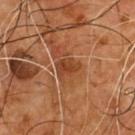{"biopsy_status": "not biopsied; imaged during a skin examination", "lighting": "cross-polarized", "image": {"source": "total-body photography crop", "field_of_view_mm": 15}, "lesion_size": {"long_diameter_mm_approx": 3.0}, "automated_metrics": {"vs_skin_darker_L": 9.0, "vs_skin_contrast_norm": 7.5, "nevus_likeness_0_100": 0, "lesion_detection_confidence_0_100": 100}, "patient": {"sex": "male", "age_approx": 55}, "site": "chest"}